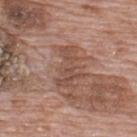A region of skin cropped from a whole-body photographic capture, roughly 15 mm wide.
From the upper back.
Longest diameter approximately 5 mm.
Automated image analysis of the tile measured a shape eccentricity near 0.9. It also reported an average lesion color of about L≈49 a*≈20 b*≈26 (CIELAB), about 8 CIELAB-L* units darker than the surrounding skin, and a normalized border contrast of about 6. It also reported an automated nevus-likeness rating near 0 out of 100 and a detector confidence of about 95 out of 100 that the crop contains a lesion.
Captured under white-light illumination.
A male patient, approximately 70 years of age.